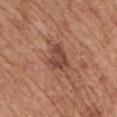Q: Is there a histopathology result?
A: total-body-photography surveillance lesion; no biopsy
Q: How was this image acquired?
A: total-body-photography crop, ~15 mm field of view
Q: What is the anatomic site?
A: the right upper arm
Q: What lighting was used for the tile?
A: white-light illumination
Q: Who is the patient?
A: female, approximately 75 years of age
Q: What did automated image analysis measure?
A: an average lesion color of about L≈45 a*≈22 b*≈27 (CIELAB), a lesion–skin lightness drop of about 10, and a lesion-to-skin contrast of about 8 (normalized; higher = more distinct); an automated nevus-likeness rating near 10 out of 100 and a lesion-detection confidence of about 100/100